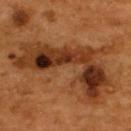  biopsy_status: not biopsied; imaged during a skin examination
  lesion_size:
    long_diameter_mm_approx: 11.5
  image:
    source: total-body photography crop
    field_of_view_mm: 15
  lighting: cross-polarized
  site: upper back
  patient:
    sex: male
    age_approx: 55